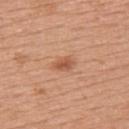– workup · catalogued during a skin exam; not biopsied
– lesion diameter · about 2.5 mm
– image · ~15 mm tile from a whole-body skin photo
– subject · female, roughly 50 years of age
– automated lesion analysis · a footprint of about 3 mm², a shape eccentricity near 0.8, and a symmetry-axis asymmetry near 0.25; an average lesion color of about L≈55 a*≈25 b*≈34 (CIELAB), roughly 10 lightness units darker than nearby skin, and a lesion-to-skin contrast of about 7 (normalized; higher = more distinct)
– tile lighting · white-light illumination
– site · the upper back